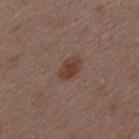The tile uses white-light illumination. On the back. A male subject, aged 48–52. A 15 mm crop from a total-body photograph taken for skin-cancer surveillance. Approximately 3 mm at its widest.